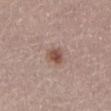{"biopsy_status": "not biopsied; imaged during a skin examination", "site": "abdomen", "patient": {"sex": "female", "age_approx": 65}, "lighting": "white-light", "image": {"source": "total-body photography crop", "field_of_view_mm": 15}, "automated_metrics": {"eccentricity": 0.5, "shape_asymmetry": 0.25, "cielab_L": 51, "cielab_a": 19, "cielab_b": 25, "vs_skin_contrast_norm": 8.0, "border_irregularity_0_10": 2.0, "color_variation_0_10": 3.5}, "lesion_size": {"long_diameter_mm_approx": 2.5}}